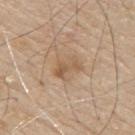| field | value |
|---|---|
| notes | no biopsy performed (imaged during a skin exam) |
| image source | ~15 mm tile from a whole-body skin photo |
| body site | the mid back |
| size | about 3 mm |
| automated lesion analysis | an area of roughly 5.5 mm² and a shape-asymmetry score of about 0.35 (0 = symmetric); a border-irregularity rating of about 4.5/10, a within-lesion color-variation index near 4/10, and radial color variation of about 1.5 |
| illumination | white-light illumination |
| subject | male, aged 78 to 82 |